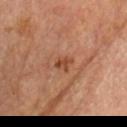| field | value |
|---|---|
| notes | total-body-photography surveillance lesion; no biopsy |
| diameter | about 2.5 mm |
| acquisition | 15 mm crop, total-body photography |
| location | the head or neck |
| tile lighting | cross-polarized |
| subject | male, aged around 55 |